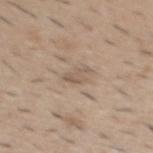Notes:
– automated lesion analysis — a shape eccentricity near 0.8 and a shape-asymmetry score of about 0.5 (0 = symmetric); a mean CIELAB color near L≈56 a*≈14 b*≈27 and a normalized lesion–skin contrast near 5.5; a border-irregularity index near 5.5/10 and a within-lesion color-variation index near 1/10; a lesion-detection confidence of about 85/100
– subject — male, aged approximately 40
– size — ≈3 mm
– imaging modality — ~15 mm crop, total-body skin-cancer survey
– lighting — white-light
– anatomic site — the mid back